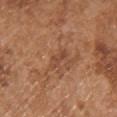Captured during whole-body skin photography for melanoma surveillance; the lesion was not biopsied. The lesion is located on the front of the torso. The patient is a male in their 70s. Automated tile analysis of the lesion measured a lesion area of about 3 mm², an outline eccentricity of about 0.95 (0 = round, 1 = elongated), and a symmetry-axis asymmetry near 0.35. The software also gave a border-irregularity index near 4/10, internal color variation of about 0 on a 0–10 scale, and a peripheral color-asymmetry measure near 0. Cropped from a whole-body photographic skin survey; the tile spans about 15 mm. Imaged with white-light lighting.